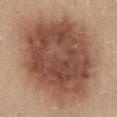The lesion was tiled from a total-body skin photograph and was not biopsied. The lesion's longest dimension is about 12.5 mm. This is a white-light tile. A female patient, in their mid- to late 40s. From the lower back. An algorithmic analysis of the crop reported a mean CIELAB color near L≈50 a*≈21 b*≈28 and a lesion–skin lightness drop of about 15. This image is a 15 mm lesion crop taken from a total-body photograph.